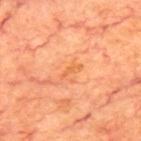| field | value |
|---|---|
| notes | imaged on a skin check; not biopsied |
| acquisition | total-body-photography crop, ~15 mm field of view |
| subject | male, aged 68 to 72 |
| site | the upper back |
| size | ~3 mm (longest diameter) |